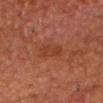follow-up: total-body-photography surveillance lesion; no biopsy
anatomic site: the front of the torso
image: ~15 mm tile from a whole-body skin photo
patient: male, aged 78–82
lesion size: about 3 mm